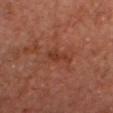notes: imaged on a skin check; not biopsied | illumination: cross-polarized | diameter: about 3 mm | subject: male, in their mid-60s | acquisition: ~15 mm tile from a whole-body skin photo | TBP lesion metrics: a lesion area of about 3 mm² and a shape-asymmetry score of about 0.4 (0 = symmetric); border irregularity of about 4 on a 0–10 scale, a color-variation rating of about 1/10, and a peripheral color-asymmetry measure near 0 | anatomic site: the head or neck.